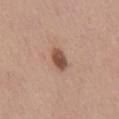notes: catalogued during a skin exam; not biopsied | automated lesion analysis: a lesion color around L≈51 a*≈21 b*≈28 in CIELAB, a lesion–skin lightness drop of about 14, and a normalized lesion–skin contrast near 9.5; a border-irregularity rating of about 2/10 | lesion diameter: about 3 mm | tile lighting: white-light | subject: male, approximately 65 years of age | imaging modality: ~15 mm tile from a whole-body skin photo | anatomic site: the mid back.